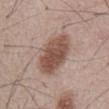workup = total-body-photography surveillance lesion; no biopsy
lesion diameter = ≈6.5 mm
subject = male, aged 53–57
acquisition = ~15 mm tile from a whole-body skin photo